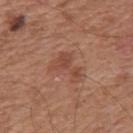Recorded during total-body skin imaging; not selected for excision or biopsy.
The lesion is located on the mid back.
A lesion tile, about 15 mm wide, cut from a 3D total-body photograph.
The subject is a male aged around 65.
About 4 mm across.
Captured under white-light illumination.